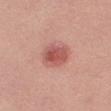No biopsy was performed on this lesion — it was imaged during a full skin examination and was not determined to be concerning. The lesion is located on the right thigh. Automated tile analysis of the lesion measured a normalized lesion–skin contrast near 7.5. And it measured a border-irregularity index near 1.5/10 and a within-lesion color-variation index near 4/10. A close-up tile cropped from a whole-body skin photograph, about 15 mm across. A female patient, in their mid-40s.